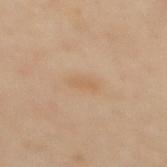Q: Is there a histopathology result?
A: imaged on a skin check; not biopsied
Q: Patient demographics?
A: female, aged 38 to 42
Q: What is the anatomic site?
A: the mid back
Q: What is the lesion's diameter?
A: ≈2.5 mm
Q: How was the tile lit?
A: cross-polarized
Q: What is the imaging modality?
A: ~15 mm tile from a whole-body skin photo